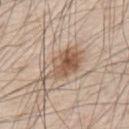Q: Patient demographics?
A: male, aged around 80
Q: Where on the body is the lesion?
A: the back
Q: How large is the lesion?
A: about 6.5 mm
Q: What is the imaging modality?
A: ~15 mm crop, total-body skin-cancer survey